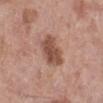This lesion was catalogued during total-body skin photography and was not selected for biopsy. A female patient, roughly 70 years of age. The lesion is on the left lower leg. The lesion's longest dimension is about 4.5 mm. A 15 mm close-up extracted from a 3D total-body photography capture.